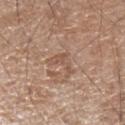Clinical impression: Recorded during total-body skin imaging; not selected for excision or biopsy. Acquisition and patient details: Imaged with white-light lighting. About 3 mm across. A 15 mm close-up tile from a total-body photography series done for melanoma screening. A male subject roughly 65 years of age. The lesion is located on the arm. Automated image analysis of the tile measured a shape eccentricity near 0.7 and a symmetry-axis asymmetry near 0.7. It also reported a nevus-likeness score of about 5/100 and lesion-presence confidence of about 100/100.